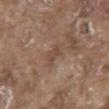follow-up: no biopsy performed (imaged during a skin exam)
subject: male, about 80 years old
image source: ~15 mm tile from a whole-body skin photo
location: the mid back